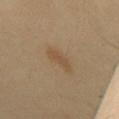follow-up — catalogued during a skin exam; not biopsied | anatomic site — the chest | lighting — cross-polarized illumination | patient — male, aged around 50 | image source — ~15 mm crop, total-body skin-cancer survey | size — about 3.5 mm.